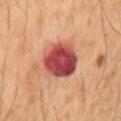Captured during whole-body skin photography for melanoma surveillance; the lesion was not biopsied. The lesion is located on the mid back. The subject is a male approximately 50 years of age. Cropped from a total-body skin-imaging series; the visible field is about 15 mm.